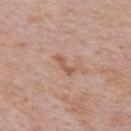This lesion was catalogued during total-body skin photography and was not selected for biopsy.
A male patient roughly 80 years of age.
Captured under white-light illumination.
Cropped from a whole-body photographic skin survey; the tile spans about 15 mm.
Longest diameter approximately 3 mm.
The lesion is on the chest.